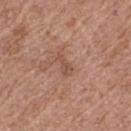A female patient aged 73–77.
Captured under white-light illumination.
Automated tile analysis of the lesion measured a mean CIELAB color near L≈51 a*≈21 b*≈28 and a lesion–skin lightness drop of about 7. The software also gave a classifier nevus-likeness of about 0/100 and lesion-presence confidence of about 100/100.
A region of skin cropped from a whole-body photographic capture, roughly 15 mm wide.
On the left thigh.
Measured at roughly 2.5 mm in maximum diameter.